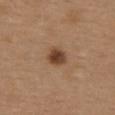Clinical summary:
On the upper back. Imaged with white-light lighting. A female patient aged approximately 40. Approximately 2.5 mm at its widest. Cropped from a whole-body photographic skin survey; the tile spans about 15 mm.